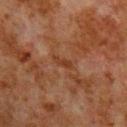Findings:
- workup — no biopsy performed (imaged during a skin exam)
- location — the chest
- subject — male, aged 78 to 82
- lesion diameter — ~2.5 mm (longest diameter)
- acquisition — total-body-photography crop, ~15 mm field of view
- TBP lesion metrics — an area of roughly 2.5 mm², an outline eccentricity of about 0.9 (0 = round, 1 = elongated), and two-axis asymmetry of about 0.35; a border-irregularity rating of about 4/10 and a within-lesion color-variation index near 0/10